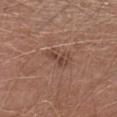biopsy status: no biopsy performed (imaged during a skin exam) | anatomic site: the left lower leg | subject: male, aged around 65 | image source: ~15 mm tile from a whole-body skin photo.